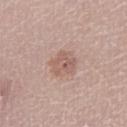Captured under white-light illumination.
Approximately 3.5 mm at its widest.
A roughly 15 mm field-of-view crop from a total-body skin photograph.
The lesion is located on the right lower leg.
The subject is a female in their 30s.